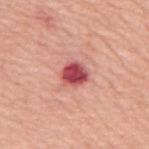This lesion was catalogued during total-body skin photography and was not selected for biopsy. Automated image analysis of the tile measured a footprint of about 6.5 mm² and an eccentricity of roughly 0.4. And it measured a mean CIELAB color near L≈52 a*≈37 b*≈24 and about 18 CIELAB-L* units darker than the surrounding skin. Captured under white-light illumination. A roughly 15 mm field-of-view crop from a total-body skin photograph. The lesion is on the back. The patient is a female aged approximately 75.